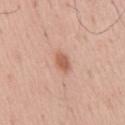biopsy_status: not biopsied; imaged during a skin examination
image:
  source: total-body photography crop
  field_of_view_mm: 15
site: mid back
lighting: white-light
lesion_size:
  long_diameter_mm_approx: 2.5
patient:
  sex: male
  age_approx: 55